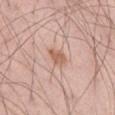notes: total-body-photography surveillance lesion; no biopsy | diameter: ≈2.5 mm | image source: ~15 mm tile from a whole-body skin photo | patient: male, aged approximately 55 | location: the right thigh.